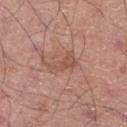Case summary:
– tile lighting: white-light illumination
– size: about 3.5 mm
– location: the right lower leg
– imaging modality: ~15 mm tile from a whole-body skin photo
– image-analysis metrics: a footprint of about 5 mm², an eccentricity of roughly 0.9, and a shape-asymmetry score of about 0.45 (0 = symmetric); a mean CIELAB color near L≈52 a*≈23 b*≈27
– subject: male, roughly 55 years of age